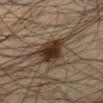Impression: Captured during whole-body skin photography for melanoma surveillance; the lesion was not biopsied. Clinical summary: Automated image analysis of the tile measured a lesion color around L≈28 a*≈14 b*≈23 in CIELAB, about 14 CIELAB-L* units darker than the surrounding skin, and a normalized border contrast of about 13.5. And it measured border irregularity of about 2.5 on a 0–10 scale, a color-variation rating of about 4/10, and a peripheral color-asymmetry measure near 1. The software also gave a nevus-likeness score of about 95/100 and lesion-presence confidence of about 100/100. On the left lower leg. The patient is a male approximately 35 years of age. This is a cross-polarized tile. A close-up tile cropped from a whole-body skin photograph, about 15 mm across.